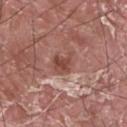| field | value |
|---|---|
| workup | no biopsy performed (imaged during a skin exam) |
| anatomic site | the back |
| patient | male, roughly 40 years of age |
| acquisition | total-body-photography crop, ~15 mm field of view |